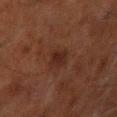Assessment: No biopsy was performed on this lesion — it was imaged during a full skin examination and was not determined to be concerning. Background: The tile uses cross-polarized illumination. The lesion is on the left arm. The patient is a male aged 58–62. Cropped from a total-body skin-imaging series; the visible field is about 15 mm. The recorded lesion diameter is about 3 mm.